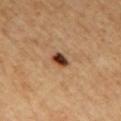{"lesion_size": {"long_diameter_mm_approx": 2.5}, "lighting": "cross-polarized", "image": {"source": "total-body photography crop", "field_of_view_mm": 15}, "site": "mid back", "patient": {"sex": "male", "age_approx": 60}}